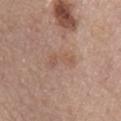Notes:
* workup: catalogued during a skin exam; not biopsied
* image source: ~15 mm crop, total-body skin-cancer survey
* site: the mid back
* lighting: white-light illumination
* patient: female, aged approximately 65
* automated metrics: a border-irregularity rating of about 5/10, a color-variation rating of about 3.5/10, and peripheral color asymmetry of about 1; an automated nevus-likeness rating near 0 out of 100 and lesion-presence confidence of about 100/100